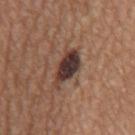image source: total-body-photography crop, ~15 mm field of view | lighting: white-light | image-analysis metrics: a lesion area of about 8.5 mm² and two-axis asymmetry of about 0.25; a lesion color around L≈35 a*≈18 b*≈21 in CIELAB, roughly 16 lightness units darker than nearby skin, and a normalized border contrast of about 14; an automated nevus-likeness rating near 10 out of 100 and a lesion-detection confidence of about 100/100 | subject: male, in their mid-60s | body site: the back | lesion diameter: ≈4.5 mm.